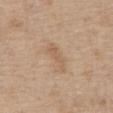Notes:
– biopsy status: no biopsy performed (imaged during a skin exam)
– body site: the abdomen
– patient: male, in their 70s
– lighting: white-light illumination
– acquisition: total-body-photography crop, ~15 mm field of view
– automated lesion analysis: an average lesion color of about L≈59 a*≈16 b*≈32 (CIELAB) and a lesion–skin lightness drop of about 7; a border-irregularity rating of about 4.5/10, a within-lesion color-variation index near 2/10, and peripheral color asymmetry of about 0.5; a nevus-likeness score of about 0/100
– diameter: ~4 mm (longest diameter)